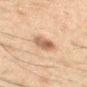automated metrics: a mean CIELAB color near L≈47 a*≈16 b*≈27 and a lesion-to-skin contrast of about 8 (normalized; higher = more distinct); a border-irregularity index near 1.5/10 and a within-lesion color-variation index near 4/10
anatomic site: the mid back
size: about 3 mm
image source: total-body-photography crop, ~15 mm field of view
patient: male, aged 48 to 52